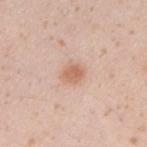Q: Is there a histopathology result?
A: no biopsy performed (imaged during a skin exam)
Q: What did automated image analysis measure?
A: a footprint of about 4 mm² and a shape-asymmetry score of about 0.2 (0 = symmetric); a border-irregularity rating of about 1.5/10, internal color variation of about 2 on a 0–10 scale, and peripheral color asymmetry of about 0.5; a nevus-likeness score of about 90/100
Q: What are the patient's age and sex?
A: female, aged 18–22
Q: How was this image acquired?
A: 15 mm crop, total-body photography
Q: How was the tile lit?
A: white-light
Q: What is the anatomic site?
A: the right forearm